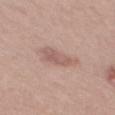This lesion was catalogued during total-body skin photography and was not selected for biopsy.
Captured under white-light illumination.
A male subject aged approximately 55.
Cropped from a whole-body photographic skin survey; the tile spans about 15 mm.
An algorithmic analysis of the crop reported a mean CIELAB color near L≈58 a*≈19 b*≈24, roughly 8 lightness units darker than nearby skin, and a lesion-to-skin contrast of about 5.5 (normalized; higher = more distinct). It also reported a border-irregularity rating of about 3.5/10, a color-variation rating of about 3/10, and a peripheral color-asymmetry measure near 1. And it measured a classifier nevus-likeness of about 5/100 and a lesion-detection confidence of about 100/100.
Measured at roughly 4 mm in maximum diameter.
The lesion is located on the mid back.